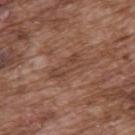The lesion was photographed on a routine skin check and not biopsied; there is no pathology result. A lesion tile, about 15 mm wide, cut from a 3D total-body photograph. Captured under white-light illumination. Measured at roughly 4 mm in maximum diameter. The lesion is on the upper back. Automated image analysis of the tile measured an area of roughly 6 mm², an eccentricity of roughly 0.9, and a symmetry-axis asymmetry near 0.35. And it measured a lesion color around L≈43 a*≈20 b*≈27 in CIELAB. It also reported a border-irregularity rating of about 4.5/10, a color-variation rating of about 2.5/10, and peripheral color asymmetry of about 0.5. The analysis additionally found an automated nevus-likeness rating near 0 out of 100 and a detector confidence of about 100 out of 100 that the crop contains a lesion. The patient is a male about 75 years old.